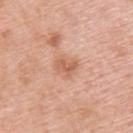Findings:
• biopsy status: no biopsy performed (imaged during a skin exam)
• body site: the upper back
• image: total-body-photography crop, ~15 mm field of view
• patient: male, aged 58–62